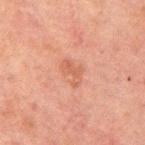Imaged during a routine full-body skin examination; the lesion was not biopsied and no histopathology is available. Located on the back. The tile uses cross-polarized illumination. A male patient, aged 58–62. Measured at roughly 3 mm in maximum diameter. A close-up tile cropped from a whole-body skin photograph, about 15 mm across.